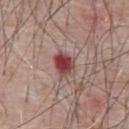| feature | finding |
|---|---|
| location | the abdomen |
| acquisition | total-body-photography crop, ~15 mm field of view |
| TBP lesion metrics | an area of roughly 6.5 mm², an outline eccentricity of about 0.65 (0 = round, 1 = elongated), and two-axis asymmetry of about 0.15; a classifier nevus-likeness of about 0/100 and a detector confidence of about 100 out of 100 that the crop contains a lesion |
| size | ≈3.5 mm |
| lighting | white-light illumination |
| patient | male, roughly 60 years of age |